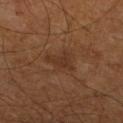Captured during whole-body skin photography for melanoma surveillance; the lesion was not biopsied.
From the left leg.
A 15 mm crop from a total-body photograph taken for skin-cancer surveillance.
The subject is a male in their mid- to late 60s.